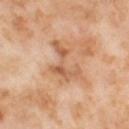This lesion was catalogued during total-body skin photography and was not selected for biopsy. The recorded lesion diameter is about 5 mm. The tile uses cross-polarized illumination. This image is a 15 mm lesion crop taken from a total-body photograph. A female patient, aged approximately 55. The lesion is located on the left thigh.